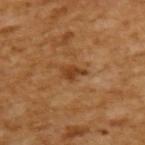{
  "biopsy_status": "not biopsied; imaged during a skin examination",
  "lighting": "cross-polarized",
  "patient": {
    "sex": "male",
    "age_approx": 65
  },
  "automated_metrics": {
    "area_mm2_approx": 3.5,
    "eccentricity": 0.8,
    "shape_asymmetry": 0.35,
    "border_irregularity_0_10": 4.0,
    "color_variation_0_10": 1.5,
    "nevus_likeness_0_100": 15,
    "lesion_detection_confidence_0_100": 100
  },
  "image": {
    "source": "total-body photography crop",
    "field_of_view_mm": 15
  },
  "lesion_size": {
    "long_diameter_mm_approx": 2.5
  }
}